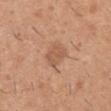notes: catalogued during a skin exam; not biopsied | patient: male, approximately 40 years of age | lighting: white-light illumination | image source: total-body-photography crop, ~15 mm field of view | site: the right upper arm | automated metrics: a mean CIELAB color near L≈56 a*≈22 b*≈33, roughly 8 lightness units darker than nearby skin, and a lesion-to-skin contrast of about 5.5 (normalized; higher = more distinct); a border-irregularity rating of about 2.5/10, a within-lesion color-variation index near 2/10, and peripheral color asymmetry of about 1; a classifier nevus-likeness of about 20/100 and a lesion-detection confidence of about 100/100.